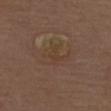Case summary:
• workup — imaged on a skin check; not biopsied
• subject — male, aged around 70
• body site — the upper back
• acquisition — ~15 mm tile from a whole-body skin photo